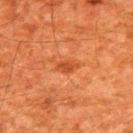Image and clinical context:
A male subject roughly 60 years of age. The lesion-visualizer software estimated a shape eccentricity near 0.85 and a symmetry-axis asymmetry near 0.2. It also reported an average lesion color of about L≈39 a*≈29 b*≈37 (CIELAB), a lesion–skin lightness drop of about 7, and a normalized lesion–skin contrast near 6.5. The analysis additionally found a border-irregularity rating of about 2/10 and radial color variation of about 0.5. It also reported a classifier nevus-likeness of about 20/100 and lesion-presence confidence of about 100/100. The tile uses cross-polarized illumination. The lesion is on the upper back. A 15 mm close-up extracted from a 3D total-body photography capture. The lesion's longest dimension is about 3 mm.